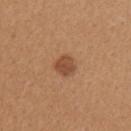Q: Was a biopsy performed?
A: total-body-photography surveillance lesion; no biopsy
Q: How was this image acquired?
A: total-body-photography crop, ~15 mm field of view
Q: What is the anatomic site?
A: the left upper arm
Q: What is the lesion's diameter?
A: ~2.5 mm (longest diameter)
Q: Who is the patient?
A: female, approximately 40 years of age
Q: Automated lesion metrics?
A: a lesion area of about 5 mm² and a shape eccentricity near 0.4; border irregularity of about 1.5 on a 0–10 scale, internal color variation of about 2 on a 0–10 scale, and radial color variation of about 0.5; an automated nevus-likeness rating near 90 out of 100 and a lesion-detection confidence of about 100/100
Q: Illumination type?
A: white-light